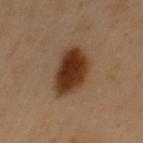Q: Was a biopsy performed?
A: total-body-photography surveillance lesion; no biopsy
Q: Illumination type?
A: cross-polarized illumination
Q: What kind of image is this?
A: ~15 mm tile from a whole-body skin photo
Q: Where on the body is the lesion?
A: the chest
Q: How large is the lesion?
A: ≈6 mm
Q: What did automated image analysis measure?
A: a border-irregularity index near 2/10 and peripheral color asymmetry of about 1.5; lesion-presence confidence of about 100/100
Q: Patient demographics?
A: male, approximately 50 years of age